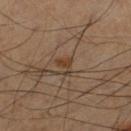Imaged during a routine full-body skin examination; the lesion was not biopsied and no histopathology is available. The lesion is on the right thigh. The subject is a male approximately 60 years of age. Approximately 2.5 mm at its widest. Cropped from a total-body skin-imaging series; the visible field is about 15 mm. The tile uses cross-polarized illumination.